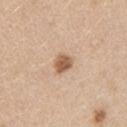workup = total-body-photography surveillance lesion; no biopsy